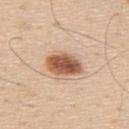Notes:
– follow-up: no biopsy performed (imaged during a skin exam)
– acquisition: ~15 mm tile from a whole-body skin photo
– body site: the upper back
– diameter: ~4.5 mm (longest diameter)
– patient: male, aged 58–62
– illumination: white-light